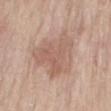| field | value |
|---|---|
| workup | total-body-photography surveillance lesion; no biopsy |
| illumination | white-light |
| site | the back |
| image source | ~15 mm tile from a whole-body skin photo |
| subject | male, aged 83 or over |